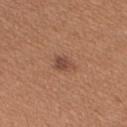Findings:
• patient · female, approximately 35 years of age
• imaging modality · ~15 mm tile from a whole-body skin photo
• lighting · white-light illumination
• location · the right upper arm
• lesion size · ~2.5 mm (longest diameter)